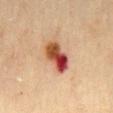Q: Was a biopsy performed?
A: no biopsy performed (imaged during a skin exam)
Q: What is the anatomic site?
A: the mid back
Q: How large is the lesion?
A: ~4.5 mm (longest diameter)
Q: What did automated image analysis measure?
A: a classifier nevus-likeness of about 0/100 and lesion-presence confidence of about 100/100
Q: Who is the patient?
A: male, aged around 45
Q: How was the tile lit?
A: cross-polarized illumination
Q: What is the imaging modality?
A: ~15 mm tile from a whole-body skin photo